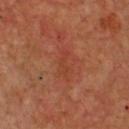Q: Is there a histopathology result?
A: no biopsy performed (imaged during a skin exam)
Q: What is the lesion's diameter?
A: ~3 mm (longest diameter)
Q: Patient demographics?
A: male, approximately 50 years of age
Q: What did automated image analysis measure?
A: an average lesion color of about L≈41 a*≈28 b*≈34 (CIELAB), about 5 CIELAB-L* units darker than the surrounding skin, and a normalized lesion–skin contrast near 4.5; an automated nevus-likeness rating near 0 out of 100 and a lesion-detection confidence of about 100/100
Q: What lighting was used for the tile?
A: cross-polarized
Q: What is the imaging modality?
A: ~15 mm tile from a whole-body skin photo
Q: Where on the body is the lesion?
A: the chest